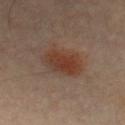Image and clinical context:
On the chest. This is a cross-polarized tile. Cropped from a whole-body photographic skin survey; the tile spans about 15 mm. The recorded lesion diameter is about 5 mm. Automated tile analysis of the lesion measured an area of roughly 12 mm², an eccentricity of roughly 0.8, and two-axis asymmetry of about 0.15. And it measured a lesion color around L≈38 a*≈20 b*≈28 in CIELAB, roughly 8 lightness units darker than nearby skin, and a normalized lesion–skin contrast near 8.5. It also reported a border-irregularity rating of about 2/10 and a color-variation rating of about 3.5/10. A male patient about 50 years old.